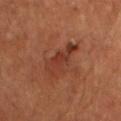Clinical impression: Recorded during total-body skin imaging; not selected for excision or biopsy. Clinical summary: On the chest. The tile uses cross-polarized illumination. A 15 mm close-up tile from a total-body photography series done for melanoma screening. Measured at roughly 5.5 mm in maximum diameter. The patient is a male in their 50s.